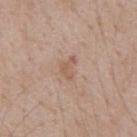Q: Was a biopsy performed?
A: imaged on a skin check; not biopsied
Q: Who is the patient?
A: male, in their 50s
Q: How was the tile lit?
A: white-light illumination
Q: How large is the lesion?
A: ~3 mm (longest diameter)
Q: What kind of image is this?
A: ~15 mm crop, total-body skin-cancer survey
Q: What is the anatomic site?
A: the mid back